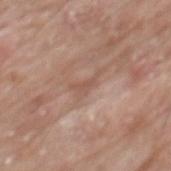The lesion was tiled from a total-body skin photograph and was not biopsied. The tile uses white-light illumination. An algorithmic analysis of the crop reported a border-irregularity rating of about 7/10, internal color variation of about 0 on a 0–10 scale, and peripheral color asymmetry of about 0. The patient is a male approximately 80 years of age. A 15 mm close-up tile from a total-body photography series done for melanoma screening. Located on the mid back. Longest diameter approximately 3.5 mm.